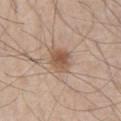- notes · no biopsy performed (imaged during a skin exam)
- location · the right thigh
- image source · ~15 mm tile from a whole-body skin photo
- illumination · white-light illumination
- subject · male, roughly 60 years of age